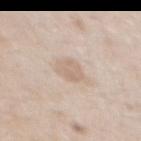Impression:
The lesion was tiled from a total-body skin photograph and was not biopsied.
Background:
A male patient, aged 53–57. Cropped from a total-body skin-imaging series; the visible field is about 15 mm. The lesion is on the chest. Longest diameter approximately 2.5 mm.